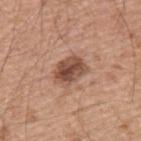The lesion was photographed on a routine skin check and not biopsied; there is no pathology result. Imaged with white-light lighting. Automated image analysis of the tile measured a lesion color around L≈49 a*≈21 b*≈28 in CIELAB and roughly 14 lightness units darker than nearby skin. It also reported a nevus-likeness score of about 70/100 and a lesion-detection confidence of about 100/100. Approximately 4 mm at its widest. The lesion is on the back. A male subject approximately 55 years of age. A close-up tile cropped from a whole-body skin photograph, about 15 mm across.